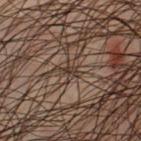The lesion was photographed on a routine skin check and not biopsied; there is no pathology result. A male patient, aged 43–47. Cropped from a whole-body photographic skin survey; the tile spans about 15 mm. Located on the chest.